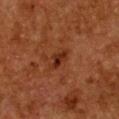notes: no biopsy performed (imaged during a skin exam)
illumination: cross-polarized
image: 15 mm crop, total-body photography
subject: female, aged around 50
anatomic site: the chest
TBP lesion metrics: a footprint of about 3.5 mm², an outline eccentricity of about 0.85 (0 = round, 1 = elongated), and two-axis asymmetry of about 0.4; an average lesion color of about L≈24 a*≈22 b*≈27 (CIELAB), roughly 8 lightness units darker than nearby skin, and a lesion-to-skin contrast of about 8.5 (normalized; higher = more distinct); a lesion-detection confidence of about 100/100